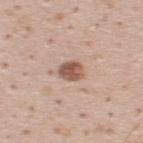Findings:
- biopsy status · imaged on a skin check; not biopsied
- subject · male, aged 33 to 37
- acquisition · ~15 mm crop, total-body skin-cancer survey
- body site · the back